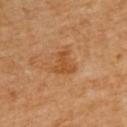Case summary:
* notes: no biopsy performed (imaged during a skin exam)
* image: total-body-photography crop, ~15 mm field of view
* lesion diameter: about 3 mm
* body site: the upper back
* subject: male, aged around 60
* tile lighting: cross-polarized illumination